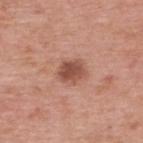Assessment: Captured during whole-body skin photography for melanoma surveillance; the lesion was not biopsied. Context: Automated image analysis of the tile measured an average lesion color of about L≈50 a*≈24 b*≈28 (CIELAB) and a normalized border contrast of about 8.5. The analysis additionally found a border-irregularity index near 1.5/10, a color-variation rating of about 3/10, and peripheral color asymmetry of about 1. Longest diameter approximately 3 mm. The lesion is on the upper back. A female patient about 40 years old. Cropped from a whole-body photographic skin survey; the tile spans about 15 mm.